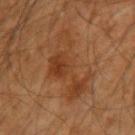| feature | finding |
|---|---|
| notes | catalogued during a skin exam; not biopsied |
| location | the left forearm |
| imaging modality | 15 mm crop, total-body photography |
| subject | male, roughly 60 years of age |
| tile lighting | cross-polarized illumination |
| size | about 8 mm |